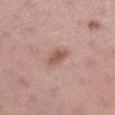Imaged during a routine full-body skin examination; the lesion was not biopsied and no histopathology is available.
The subject is a female aged approximately 25.
The lesion is on the leg.
A 15 mm close-up extracted from a 3D total-body photography capture.
The tile uses white-light illumination.